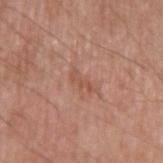The lesion was photographed on a routine skin check and not biopsied; there is no pathology result. A male patient, aged 63 to 67. A lesion tile, about 15 mm wide, cut from a 3D total-body photograph. Approximately 2.5 mm at its widest. Captured under white-light illumination. From the left upper arm.